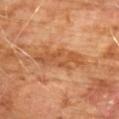Clinical impression: Recorded during total-body skin imaging; not selected for excision or biopsy. Clinical summary: The lesion's longest dimension is about 7 mm. A male patient aged 58 to 62. The lesion is located on the chest. A 15 mm crop from a total-body photograph taken for skin-cancer surveillance. Captured under cross-polarized illumination.